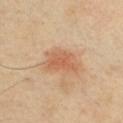Clinical impression:
This lesion was catalogued during total-body skin photography and was not selected for biopsy.
Background:
A 15 mm close-up tile from a total-body photography series done for melanoma screening. A male subject, aged 38–42. From the chest.